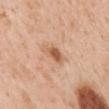{
  "biopsy_status": "not biopsied; imaged during a skin examination",
  "site": "back",
  "lighting": "white-light",
  "patient": {
    "sex": "female",
    "age_approx": 50
  },
  "image": {
    "source": "total-body photography crop",
    "field_of_view_mm": 15
  }
}